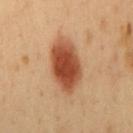– workup — total-body-photography surveillance lesion; no biopsy
– subject — male, aged around 50
– anatomic site — the mid back
– automated lesion analysis — a footprint of about 18 mm², an outline eccentricity of about 0.85 (0 = round, 1 = elongated), and a symmetry-axis asymmetry near 0.2; a within-lesion color-variation index near 5.5/10 and peripheral color asymmetry of about 1.5
– lighting — cross-polarized illumination
– image source — ~15 mm crop, total-body skin-cancer survey
– diameter — ~7 mm (longest diameter)